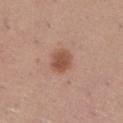Notes:
- notes — no biopsy performed (imaged during a skin exam)
- subject — female, aged 28 to 32
- image — total-body-photography crop, ~15 mm field of view
- tile lighting — white-light
- automated metrics — a border-irregularity rating of about 1.5/10, internal color variation of about 2.5 on a 0–10 scale, and peripheral color asymmetry of about 0.5; a classifier nevus-likeness of about 95/100 and lesion-presence confidence of about 100/100
- anatomic site — the leg